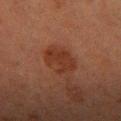| field | value |
|---|---|
| follow-up | no biopsy performed (imaged during a skin exam) |
| illumination | cross-polarized illumination |
| acquisition | total-body-photography crop, ~15 mm field of view |
| anatomic site | the right forearm |
| lesion diameter | ≈4 mm |
| patient | female, aged 58–62 |